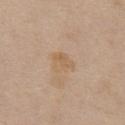<tbp_lesion>
  <biopsy_status>not biopsied; imaged during a skin examination</biopsy_status>
  <patient>
    <sex>female</sex>
    <age_approx>70</age_approx>
  </patient>
  <image>
    <source>total-body photography crop</source>
    <field_of_view_mm>15</field_of_view_mm>
  </image>
  <site>chest</site>
  <lesion_size>
    <long_diameter_mm_approx>2.5</long_diameter_mm_approx>
  </lesion_size>
  <lighting>white-light</lighting>
</tbp_lesion>